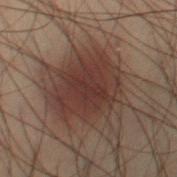Imaged during a routine full-body skin examination; the lesion was not biopsied and no histopathology is available. Imaged with cross-polarized lighting. A region of skin cropped from a whole-body photographic capture, roughly 15 mm wide. The patient is a male approximately 55 years of age. The lesion is located on the right thigh.